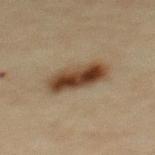Automated image analysis of the tile measured roughly 14 lightness units darker than nearby skin and a normalized border contrast of about 12. The analysis additionally found a border-irregularity index near 2/10 and a peripheral color-asymmetry measure near 2.5. The software also gave an automated nevus-likeness rating near 100 out of 100 and a lesion-detection confidence of about 100/100.
A male patient about 45 years old.
The lesion is located on the upper back.
Captured under cross-polarized illumination.
Cropped from a total-body skin-imaging series; the visible field is about 15 mm.
The lesion's longest dimension is about 6 mm.